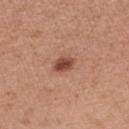workup: imaged on a skin check; not biopsied | size: ≈3 mm | image-analysis metrics: a footprint of about 4.5 mm² and a symmetry-axis asymmetry near 0.2; a lesion color around L≈47 a*≈24 b*≈29 in CIELAB, a lesion–skin lightness drop of about 13, and a lesion-to-skin contrast of about 9.5 (normalized; higher = more distinct); a border-irregularity index near 1.5/10 and internal color variation of about 3 on a 0–10 scale | lighting: white-light illumination | anatomic site: the left upper arm | subject: female, aged 28 to 32 | imaging modality: ~15 mm tile from a whole-body skin photo.